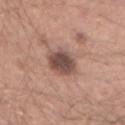biopsy status: imaged on a skin check; not biopsied
lighting: white-light
location: the right forearm
patient: male, roughly 30 years of age
lesion size: ≈4 mm
image: 15 mm crop, total-body photography